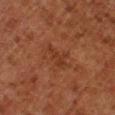biopsy_status: not biopsied; imaged during a skin examination
site: leg
patient:
  sex: male
  age_approx: 80
lighting: cross-polarized
image:
  source: total-body photography crop
  field_of_view_mm: 15
lesion_size:
  long_diameter_mm_approx: 4.0
automated_metrics:
  cielab_L: 27
  cielab_a: 20
  cielab_b: 26
  vs_skin_contrast_norm: 5.0
  color_variation_0_10: 1.0
  peripheral_color_asymmetry: 0.0
  nevus_likeness_0_100: 0
  lesion_detection_confidence_0_100: 100